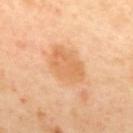Impression: The lesion was photographed on a routine skin check and not biopsied; there is no pathology result. Acquisition and patient details: From the mid back. A male subject, in their mid-60s. A region of skin cropped from a whole-body photographic capture, roughly 15 mm wide. Longest diameter approximately 5 mm. Imaged with cross-polarized lighting.